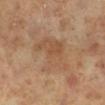Clinical impression: Recorded during total-body skin imaging; not selected for excision or biopsy. Clinical summary: Automated image analysis of the tile measured an area of roughly 15 mm² and an outline eccentricity of about 0.8 (0 = round, 1 = elongated). And it measured a lesion color around L≈53 a*≈19 b*≈33 in CIELAB, a lesion–skin lightness drop of about 7, and a lesion-to-skin contrast of about 5 (normalized; higher = more distinct). On the leg. This image is a 15 mm lesion crop taken from a total-body photograph. Imaged with cross-polarized lighting. A female subject aged 68 to 72.